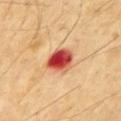follow-up: no biopsy performed (imaged during a skin exam)
size: about 3.5 mm
site: the chest
image source: 15 mm crop, total-body photography
patient: male, aged around 70
lighting: cross-polarized
image-analysis metrics: a lesion color around L≈50 a*≈36 b*≈34 in CIELAB